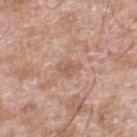Q: Was this lesion biopsied?
A: imaged on a skin check; not biopsied
Q: What are the patient's age and sex?
A: male, in their 60s
Q: How was the tile lit?
A: white-light illumination
Q: What is the imaging modality?
A: ~15 mm crop, total-body skin-cancer survey
Q: Lesion location?
A: the right lower leg
Q: How large is the lesion?
A: ≈3 mm